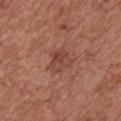  patient:
    sex: male
    age_approx: 75
  site: chest
  image:
    source: total-body photography crop
    field_of_view_mm: 15